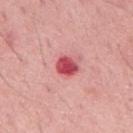biopsy status = catalogued during a skin exam; not biopsied | image = ~15 mm crop, total-body skin-cancer survey | body site = the mid back | subject = male, aged approximately 65 | automated metrics = a footprint of about 4.5 mm², a shape eccentricity near 0.5, and a symmetry-axis asymmetry near 0.25; internal color variation of about 3.5 on a 0–10 scale and radial color variation of about 1; a classifier nevus-likeness of about 0/100 and a detector confidence of about 100 out of 100 that the crop contains a lesion | illumination = white-light illumination | lesion diameter = about 2.5 mm.